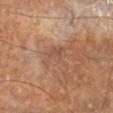Assessment:
Recorded during total-body skin imaging; not selected for excision or biopsy.
Image and clinical context:
The lesion is on the right lower leg. This image is a 15 mm lesion crop taken from a total-body photograph. Automated tile analysis of the lesion measured a lesion area of about 7 mm² and a shape eccentricity near 0.65. The analysis additionally found about 6 CIELAB-L* units darker than the surrounding skin and a normalized border contrast of about 5. This is a cross-polarized tile. Longest diameter approximately 3.5 mm. A male subject, roughly 60 years of age.